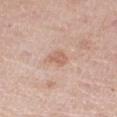The lesion was photographed on a routine skin check and not biopsied; there is no pathology result. About 2.5 mm across. The tile uses white-light illumination. A 15 mm crop from a total-body photograph taken for skin-cancer surveillance. The patient is a female aged 58–62. Located on the right thigh.